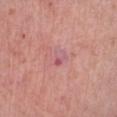Clinical impression: The lesion was photographed on a routine skin check and not biopsied; there is no pathology result. Image and clinical context: This image is a 15 mm lesion crop taken from a total-body photograph. The subject is a female in their mid- to late 50s. The lesion is located on the left lower leg. The lesion's longest dimension is about 2.5 mm.